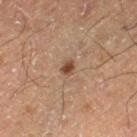The lesion was photographed on a routine skin check and not biopsied; there is no pathology result.
On the left thigh.
Approximately 2 mm at its widest.
Imaged with cross-polarized lighting.
Cropped from a whole-body photographic skin survey; the tile spans about 15 mm.
The patient is a male roughly 60 years of age.